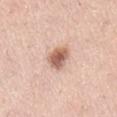Imaged during a routine full-body skin examination; the lesion was not biopsied and no histopathology is available.
The lesion is located on the lower back.
A roughly 15 mm field-of-view crop from a total-body skin photograph.
A female subject aged 38–42.
The lesion-visualizer software estimated a lesion area of about 6 mm², a shape eccentricity near 0.45, and two-axis asymmetry of about 0.2. It also reported a mean CIELAB color near L≈61 a*≈22 b*≈28, roughly 16 lightness units darker than nearby skin, and a lesion-to-skin contrast of about 9.5 (normalized; higher = more distinct). The analysis additionally found a border-irregularity rating of about 2/10, a color-variation rating of about 4.5/10, and peripheral color asymmetry of about 1.5.